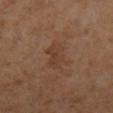Imaged during a routine full-body skin examination; the lesion was not biopsied and no histopathology is available.